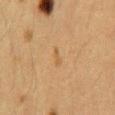workup: imaged on a skin check; not biopsied | anatomic site: the back | tile lighting: cross-polarized | image-analysis metrics: an area of roughly 1.5 mm², an eccentricity of roughly 0.95, and two-axis asymmetry of about 0.55; an average lesion color of about L≈47 a*≈16 b*≈34 (CIELAB), about 5 CIELAB-L* units darker than the surrounding skin, and a lesion-to-skin contrast of about 5 (normalized; higher = more distinct); border irregularity of about 5.5 on a 0–10 scale, a color-variation rating of about 0/10, and radial color variation of about 0; a classifier nevus-likeness of about 0/100 and a lesion-detection confidence of about 100/100 | subject: female, about 40 years old | lesion size: ~2 mm (longest diameter) | imaging modality: 15 mm crop, total-body photography.